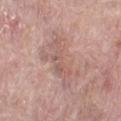Assessment: The lesion was tiled from a total-body skin photograph and was not biopsied. Clinical summary: The lesion's longest dimension is about 7 mm. A female subject approximately 60 years of age. From the left lower leg. Imaged with white-light lighting. This image is a 15 mm lesion crop taken from a total-body photograph.